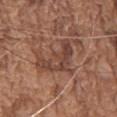Captured during whole-body skin photography for melanoma surveillance; the lesion was not biopsied. The lesion's longest dimension is about 7 mm. On the chest. This is a white-light tile. A male patient, in their mid- to late 70s. A close-up tile cropped from a whole-body skin photograph, about 15 mm across.